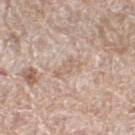notes — imaged on a skin check; not biopsied | body site — the right thigh | tile lighting — white-light | image source — total-body-photography crop, ~15 mm field of view | size — ~3 mm (longest diameter) | subject — female, approximately 60 years of age | automated lesion analysis — a border-irregularity rating of about 5/10, a within-lesion color-variation index near 0.5/10, and radial color variation of about 0.